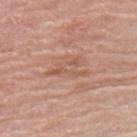{
  "lighting": "white-light",
  "patient": {
    "sex": "female",
    "age_approx": 60
  },
  "image": {
    "source": "total-body photography crop",
    "field_of_view_mm": 15
  },
  "automated_metrics": {
    "area_mm2_approx": 6.5,
    "shape_asymmetry": 0.55,
    "vs_skin_darker_L": 7.0,
    "vs_skin_contrast_norm": 5.5,
    "border_irregularity_0_10": 7.0,
    "color_variation_0_10": 3.5,
    "peripheral_color_asymmetry": 1.0
  },
  "site": "right upper arm"
}